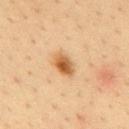This lesion was catalogued during total-body skin photography and was not selected for biopsy.
Imaged with cross-polarized lighting.
Measured at roughly 3 mm in maximum diameter.
A 15 mm close-up tile from a total-body photography series done for melanoma screening.
From the mid back.
A male patient, aged around 35.
Automated image analysis of the tile measured a lesion area of about 5.5 mm², an outline eccentricity of about 0.75 (0 = round, 1 = elongated), and a shape-asymmetry score of about 0.15 (0 = symmetric). And it measured a mean CIELAB color near L≈55 a*≈21 b*≈39, roughly 13 lightness units darker than nearby skin, and a normalized border contrast of about 9.5. The analysis additionally found an automated nevus-likeness rating near 100 out of 100 and a lesion-detection confidence of about 100/100.